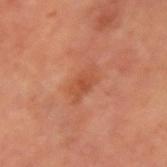Captured during whole-body skin photography for melanoma surveillance; the lesion was not biopsied. From the left upper arm. The patient is a male aged around 65. The tile uses cross-polarized illumination. A roughly 15 mm field-of-view crop from a total-body skin photograph. The recorded lesion diameter is about 4 mm.